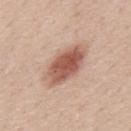Clinical impression: No biopsy was performed on this lesion — it was imaged during a full skin examination and was not determined to be concerning. Background: A male patient in their 60s. A lesion tile, about 15 mm wide, cut from a 3D total-body photograph. From the mid back. This is a white-light tile.